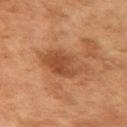Impression:
Recorded during total-body skin imaging; not selected for excision or biopsy.
Acquisition and patient details:
The lesion is located on the left upper arm. Longest diameter approximately 5.5 mm. A female subject, in their 60s. This image is a 15 mm lesion crop taken from a total-body photograph. Automated image analysis of the tile measured a lesion area of about 14 mm² and an eccentricity of roughly 0.75. The analysis additionally found a mean CIELAB color near L≈43 a*≈22 b*≈32, a lesion–skin lightness drop of about 9, and a lesion-to-skin contrast of about 7 (normalized; higher = more distinct). The analysis additionally found a border-irregularity index near 2.5/10 and a peripheral color-asymmetry measure near 1. It also reported a classifier nevus-likeness of about 0/100 and a lesion-detection confidence of about 100/100.